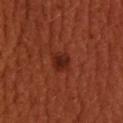Imaged during a routine full-body skin examination; the lesion was not biopsied and no histopathology is available.